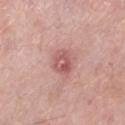Case summary:
- follow-up — no biopsy performed (imaged during a skin exam)